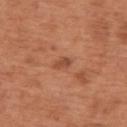A roughly 15 mm field-of-view crop from a total-body skin photograph.
The lesion is located on the back.
A male subject aged 63 to 67.